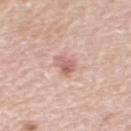The lesion was photographed on a routine skin check and not biopsied; there is no pathology result. The total-body-photography lesion software estimated a footprint of about 4.5 mm², a shape eccentricity near 0.5, and two-axis asymmetry of about 0.35. It also reported an automated nevus-likeness rating near 10 out of 100. Cropped from a total-body skin-imaging series; the visible field is about 15 mm. The lesion is located on the left upper arm. Longest diameter approximately 2.5 mm. A female subject, roughly 50 years of age.